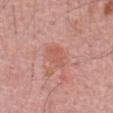<case>
<biopsy_status>not biopsied; imaged during a skin examination</biopsy_status>
<automated_metrics>
  <area_mm2_approx>6.0</area_mm2_approx>
  <eccentricity>0.7</eccentricity>
  <shape_asymmetry>0.25</shape_asymmetry>
  <cielab_L>58</cielab_L>
  <cielab_a>27</cielab_a>
  <cielab_b>29</cielab_b>
  <vs_skin_darker_L>6.0</vs_skin_darker_L>
  <border_irregularity_0_10>3.0</border_irregularity_0_10>
  <color_variation_0_10>2.0</color_variation_0_10>
  <peripheral_color_asymmetry>1.0</peripheral_color_asymmetry>
  <nevus_likeness_0_100>0</nevus_likeness_0_100>
</automated_metrics>
<image>
  <source>total-body photography crop</source>
  <field_of_view_mm>15</field_of_view_mm>
</image>
<patient>
  <sex>male</sex>
  <age_approx>50</age_approx>
</patient>
<site>abdomen</site>
</case>